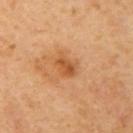<case>
<biopsy_status>not biopsied; imaged during a skin examination</biopsy_status>
<lesion_size>
  <long_diameter_mm_approx>3.5</long_diameter_mm_approx>
</lesion_size>
<automated_metrics>
  <eccentricity>0.75</eccentricity>
  <shape_asymmetry>0.3</shape_asymmetry>
  <cielab_L>54</cielab_L>
  <cielab_a>26</cielab_a>
  <cielab_b>41</cielab_b>
  <vs_skin_darker_L>10.0</vs_skin_darker_L>
  <vs_skin_contrast_norm>7.0</vs_skin_contrast_norm>
  <nevus_likeness_0_100>15</nevus_likeness_0_100>
</automated_metrics>
<patient>
  <sex>female</sex>
  <age_approx>40</age_approx>
</patient>
<image>
  <source>total-body photography crop</source>
  <field_of_view_mm>15</field_of_view_mm>
</image>
<site>left upper arm</site>
</case>